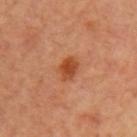Assessment: Part of a total-body skin-imaging series; this lesion was reviewed on a skin check and was not flagged for biopsy. Image and clinical context: The patient is a female approximately 40 years of age. Located on the left upper arm. Cropped from a total-body skin-imaging series; the visible field is about 15 mm. Approximately 3 mm at its widest. This is a cross-polarized tile.